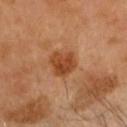Assessment:
This lesion was catalogued during total-body skin photography and was not selected for biopsy.
Context:
The lesion-visualizer software estimated an area of roughly 8.5 mm², a shape eccentricity near 0.5, and two-axis asymmetry of about 0.25. It also reported internal color variation of about 4 on a 0–10 scale. The subject is a male aged approximately 65. A region of skin cropped from a whole-body photographic capture, roughly 15 mm wide. Imaged with cross-polarized lighting. From the head or neck.